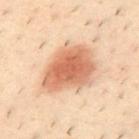A close-up tile cropped from a whole-body skin photograph, about 15 mm across. Automated image analysis of the tile measured an outline eccentricity of about 0.7 (0 = round, 1 = elongated) and a shape-asymmetry score of about 0.25 (0 = symmetric). Located on the upper back. A male patient aged around 40.